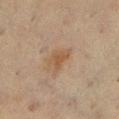{"biopsy_status": "not biopsied; imaged during a skin examination", "lesion_size": {"long_diameter_mm_approx": 3.0}, "image": {"source": "total-body photography crop", "field_of_view_mm": 15}, "site": "left lower leg", "patient": {"sex": "female", "age_approx": 55}, "lighting": "cross-polarized", "automated_metrics": {"area_mm2_approx": 4.5, "eccentricity": 0.8, "shape_asymmetry": 0.4, "cielab_L": 42, "cielab_a": 14, "cielab_b": 28, "vs_skin_darker_L": 6.0, "vs_skin_contrast_norm": 6.5, "border_irregularity_0_10": 4.5, "peripheral_color_asymmetry": 0.5, "nevus_likeness_0_100": 25, "lesion_detection_confidence_0_100": 100}}